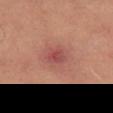{
  "biopsy_status": "not biopsied; imaged during a skin examination",
  "site": "right thigh",
  "lighting": "cross-polarized",
  "lesion_size": {
    "long_diameter_mm_approx": 2.5
  },
  "automated_metrics": {
    "area_mm2_approx": 4.0,
    "eccentricity": 0.55,
    "shape_asymmetry": 0.2,
    "border_irregularity_0_10": 1.5,
    "peripheral_color_asymmetry": 1.5,
    "nevus_likeness_0_100": 0,
    "lesion_detection_confidence_0_100": 100
  },
  "patient": {
    "age_approx": 65
  },
  "image": {
    "source": "total-body photography crop",
    "field_of_view_mm": 15
  }
}